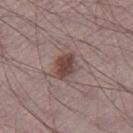Assessment:
The lesion was tiled from a total-body skin photograph and was not biopsied.
Context:
Approximately 3.5 mm at its widest. Located on the leg. Captured under white-light illumination. A male subject in their 70s. Automated tile analysis of the lesion measured a lesion color around L≈44 a*≈17 b*≈20 in CIELAB and a normalized lesion–skin contrast near 8.5. It also reported border irregularity of about 2 on a 0–10 scale and internal color variation of about 4 on a 0–10 scale. It also reported an automated nevus-likeness rating near 90 out of 100 and a lesion-detection confidence of about 100/100. Cropped from a total-body skin-imaging series; the visible field is about 15 mm.